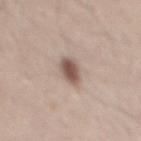Q: Is there a histopathology result?
A: catalogued during a skin exam; not biopsied
Q: What are the patient's age and sex?
A: male, aged approximately 35
Q: What is the anatomic site?
A: the mid back
Q: What lighting was used for the tile?
A: white-light illumination
Q: What is the imaging modality?
A: ~15 mm tile from a whole-body skin photo